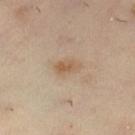Impression: Part of a total-body skin-imaging series; this lesion was reviewed on a skin check and was not flagged for biopsy. Background: An algorithmic analysis of the crop reported border irregularity of about 2.5 on a 0–10 scale and peripheral color asymmetry of about 1. The subject is a female approximately 45 years of age. About 3 mm across. The tile uses cross-polarized illumination. A region of skin cropped from a whole-body photographic capture, roughly 15 mm wide. The lesion is located on the left lower leg.